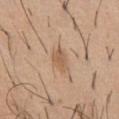Findings:
* notes — no biopsy performed (imaged during a skin exam)
* size — ~3 mm (longest diameter)
* image — 15 mm crop, total-body photography
* site — the chest
* patient — male, aged 38–42
* tile lighting — white-light